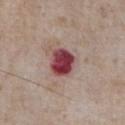Clinical impression: No biopsy was performed on this lesion — it was imaged during a full skin examination and was not determined to be concerning. Clinical summary: Longest diameter approximately 3.5 mm. Located on the chest. A male patient, aged 73 to 77. Captured under white-light illumination. This image is a 15 mm lesion crop taken from a total-body photograph.